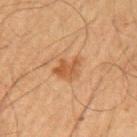Q: Was a biopsy performed?
A: no biopsy performed (imaged during a skin exam)
Q: What is the imaging modality?
A: ~15 mm tile from a whole-body skin photo
Q: Where on the body is the lesion?
A: the left upper arm
Q: Who is the patient?
A: male, aged approximately 65